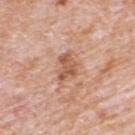Captured during whole-body skin photography for melanoma surveillance; the lesion was not biopsied.
On the back.
A region of skin cropped from a whole-body photographic capture, roughly 15 mm wide.
Automated tile analysis of the lesion measured a lesion color around L≈57 a*≈23 b*≈32 in CIELAB, roughly 11 lightness units darker than nearby skin, and a normalized border contrast of about 7.5. It also reported a border-irregularity index near 4/10 and radial color variation of about 1. And it measured a nevus-likeness score of about 0/100.
The subject is a male aged 78 to 82.